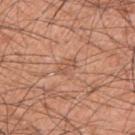Q: Was this lesion biopsied?
A: imaged on a skin check; not biopsied
Q: What is the anatomic site?
A: the left upper arm
Q: How large is the lesion?
A: about 2.5 mm
Q: What kind of image is this?
A: total-body-photography crop, ~15 mm field of view
Q: Who is the patient?
A: male, roughly 60 years of age
Q: How was the tile lit?
A: white-light illumination